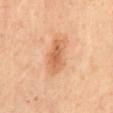{"lesion_size": {"long_diameter_mm_approx": 5.0}, "lighting": "cross-polarized", "site": "back", "image": {"source": "total-body photography crop", "field_of_view_mm": 15}, "patient": {"sex": "female", "age_approx": 45}, "automated_metrics": {"cielab_L": 62, "cielab_a": 23, "cielab_b": 37, "vs_skin_darker_L": 10.0, "vs_skin_contrast_norm": 7.0, "border_irregularity_0_10": 3.0, "peripheral_color_asymmetry": 1.0}}